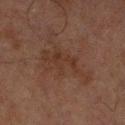Findings:
- notes: imaged on a skin check; not biopsied
- subject: male, roughly 65 years of age
- site: the left lower leg
- lesion size: ≈6 mm
- imaging modality: ~15 mm crop, total-body skin-cancer survey
- lighting: cross-polarized
- automated lesion analysis: a mean CIELAB color near L≈26 a*≈15 b*≈21, about 4 CIELAB-L* units darker than the surrounding skin, and a normalized border contrast of about 5.5; a color-variation rating of about 2.5/10 and peripheral color asymmetry of about 0.5